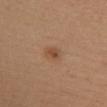* workup: total-body-photography surveillance lesion; no biopsy
* size: ~2.5 mm (longest diameter)
* illumination: white-light
* image source: 15 mm crop, total-body photography
* patient: female, aged approximately 45
* anatomic site: the upper back